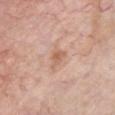{"biopsy_status": "not biopsied; imaged during a skin examination", "lighting": "white-light", "site": "chest", "patient": {"sex": "male", "age_approx": 60}, "lesion_size": {"long_diameter_mm_approx": 3.0}, "image": {"source": "total-body photography crop", "field_of_view_mm": 15}}